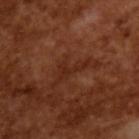{
  "lesion_size": {
    "long_diameter_mm_approx": 4.0
  },
  "image": {
    "source": "total-body photography crop",
    "field_of_view_mm": 15
  },
  "lighting": "cross-polarized",
  "patient": {
    "sex": "male",
    "age_approx": 65
  }
}